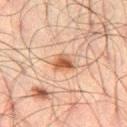image: ~15 mm tile from a whole-body skin photo
anatomic site: the left thigh
patient: male, about 50 years old
tile lighting: cross-polarized illumination
image-analysis metrics: a footprint of about 4 mm², an eccentricity of roughly 0.65, and a symmetry-axis asymmetry near 0.35; a color-variation rating of about 3.5/10